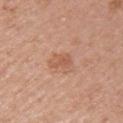Captured during whole-body skin photography for melanoma surveillance; the lesion was not biopsied.
Cropped from a whole-body photographic skin survey; the tile spans about 15 mm.
From the left upper arm.
The tile uses white-light illumination.
A female subject, approximately 55 years of age.